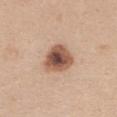Clinical impression:
The lesion was tiled from a total-body skin photograph and was not biopsied.
Background:
A female subject, aged 18–22. The recorded lesion diameter is about 4 mm. A lesion tile, about 15 mm wide, cut from a 3D total-body photograph. From the chest. This is a white-light tile.